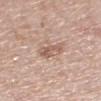No biopsy was performed on this lesion — it was imaged during a full skin examination and was not determined to be concerning.
Approximately 3.5 mm at its widest.
The lesion is located on the left lower leg.
A 15 mm close-up tile from a total-body photography series done for melanoma screening.
A female subject about 65 years old.
The lesion-visualizer software estimated a mean CIELAB color near L≈58 a*≈19 b*≈26 and a lesion-to-skin contrast of about 6.5 (normalized; higher = more distinct). It also reported a border-irregularity rating of about 3.5/10, a color-variation rating of about 2.5/10, and peripheral color asymmetry of about 1.